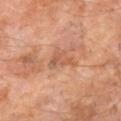biopsy status=catalogued during a skin exam; not biopsied
image=15 mm crop, total-body photography
automated metrics=a mean CIELAB color near L≈52 a*≈22 b*≈31 and roughly 8 lightness units darker than nearby skin; a within-lesion color-variation index near 3/10 and a peripheral color-asymmetry measure near 1; a nevus-likeness score of about 0/100
diameter=≈3 mm
patient=male, aged around 60
tile lighting=cross-polarized illumination
site=the right lower leg